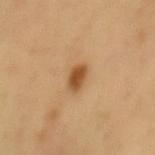Assessment:
Recorded during total-body skin imaging; not selected for excision or biopsy.
Clinical summary:
The lesion-visualizer software estimated an area of roughly 4.5 mm², an outline eccentricity of about 0.75 (0 = round, 1 = elongated), and a symmetry-axis asymmetry near 0.2. The analysis additionally found a border-irregularity index near 2/10 and internal color variation of about 2.5 on a 0–10 scale. A male patient, aged around 50. Measured at roughly 3 mm in maximum diameter. Cropped from a total-body skin-imaging series; the visible field is about 15 mm. On the mid back.